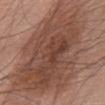Part of a total-body skin-imaging series; this lesion was reviewed on a skin check and was not flagged for biopsy. The total-body-photography lesion software estimated an average lesion color of about L≈47 a*≈20 b*≈26 (CIELAB) and a lesion–skin lightness drop of about 11. And it measured internal color variation of about 5.5 on a 0–10 scale and radial color variation of about 2. The analysis additionally found a classifier nevus-likeness of about 25/100 and lesion-presence confidence of about 100/100. The subject is a male roughly 75 years of age. On the abdomen. A roughly 15 mm field-of-view crop from a total-body skin photograph.